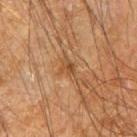The lesion-visualizer software estimated a lesion area of about 4 mm² and a symmetry-axis asymmetry near 0.3. It also reported roughly 7 lightness units darker than nearby skin and a lesion-to-skin contrast of about 6 (normalized; higher = more distinct). And it measured border irregularity of about 4 on a 0–10 scale and peripheral color asymmetry of about 1.5. And it measured a nevus-likeness score of about 0/100 and a detector confidence of about 95 out of 100 that the crop contains a lesion. Located on the right upper arm. The patient is a male aged 63 to 67. Captured under cross-polarized illumination. Cropped from a total-body skin-imaging series; the visible field is about 15 mm.